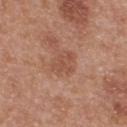Captured during whole-body skin photography for melanoma surveillance; the lesion was not biopsied.